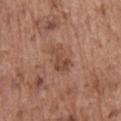This lesion was catalogued during total-body skin photography and was not selected for biopsy. A close-up tile cropped from a whole-body skin photograph, about 15 mm across. Captured under white-light illumination. Automated tile analysis of the lesion measured border irregularity of about 5.5 on a 0–10 scale, a within-lesion color-variation index near 2/10, and peripheral color asymmetry of about 1. A male subject about 75 years old. The lesion's longest dimension is about 3.5 mm. The lesion is on the front of the torso.